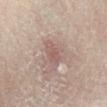imaging modality: ~15 mm tile from a whole-body skin photo
site: the right lower leg
lesion diameter: ≈2.5 mm
subject: male, aged 73 to 77
automated lesion analysis: an area of roughly 4.5 mm², an eccentricity of roughly 0.65, and a symmetry-axis asymmetry near 0.25; a border-irregularity rating of about 2.5/10, a color-variation rating of about 1.5/10, and a peripheral color-asymmetry measure near 0.5; a nevus-likeness score of about 0/100
lighting: cross-polarized illumination